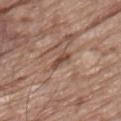- biopsy status: total-body-photography surveillance lesion; no biopsy
- image-analysis metrics: a normalized lesion–skin contrast near 7.5
- diameter: about 3 mm
- subject: male, roughly 80 years of age
- anatomic site: the lower back
- image: ~15 mm crop, total-body skin-cancer survey